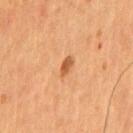The lesion was tiled from a total-body skin photograph and was not biopsied.
The lesion is located on the chest.
A roughly 15 mm field-of-view crop from a total-body skin photograph.
A male subject about 55 years old.